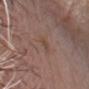biopsy_status: not biopsied; imaged during a skin examination
automated_metrics:
  cielab_L: 46
  cielab_a: 18
  cielab_b: 26
  vs_skin_darker_L: 5.0
  vs_skin_contrast_norm: 5.0
  lesion_detection_confidence_0_100: 100
patient:
  sex: male
  age_approx: 65
site: head or neck
lighting: white-light
lesion_size:
  long_diameter_mm_approx: 3.0
image:
  source: total-body photography crop
  field_of_view_mm: 15